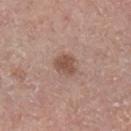The lesion was tiled from a total-body skin photograph and was not biopsied.
About 2.5 mm across.
Captured under white-light illumination.
A male patient in their mid-50s.
The total-body-photography lesion software estimated a lesion area of about 4.5 mm² and two-axis asymmetry of about 0.3. The analysis additionally found a within-lesion color-variation index near 1.5/10.
The lesion is on the left lower leg.
A lesion tile, about 15 mm wide, cut from a 3D total-body photograph.